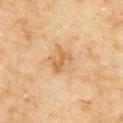Acquisition and patient details:
A 15 mm close-up tile from a total-body photography series done for melanoma screening. On the upper back. The lesion-visualizer software estimated a footprint of about 4 mm² and two-axis asymmetry of about 0.3. The analysis additionally found border irregularity of about 4 on a 0–10 scale and a within-lesion color-variation index near 1/10. The software also gave lesion-presence confidence of about 100/100. A female patient aged approximately 60. Captured under cross-polarized illumination.